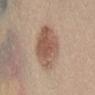Recorded during total-body skin imaging; not selected for excision or biopsy. The lesion's longest dimension is about 5 mm. A female subject, in their 40s. Cropped from a whole-body photographic skin survey; the tile spans about 15 mm. Imaged with white-light lighting. On the lower back.